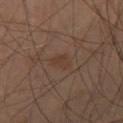– workup: total-body-photography surveillance lesion; no biopsy
– image source: ~15 mm crop, total-body skin-cancer survey
– subject: male, about 70 years old
– lighting: cross-polarized
– anatomic site: the leg